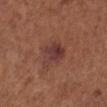Part of a total-body skin-imaging series; this lesion was reviewed on a skin check and was not flagged for biopsy. From the left lower leg. A female subject, aged 53–57. A 15 mm crop from a total-body photograph taken for skin-cancer surveillance.